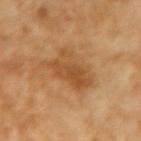Clinical impression: This lesion was catalogued during total-body skin photography and was not selected for biopsy. Background: A female patient, in their 70s. A roughly 15 mm field-of-view crop from a total-body skin photograph. Measured at roughly 7 mm in maximum diameter. Imaged with cross-polarized lighting. From the left forearm.